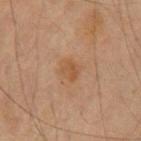biopsy status = total-body-photography surveillance lesion; no biopsy | illumination = cross-polarized illumination | anatomic site = the arm | subject = male, aged around 60 | acquisition = 15 mm crop, total-body photography.